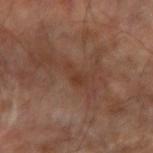<record>
  <biopsy_status>not biopsied; imaged during a skin examination</biopsy_status>
  <lighting>cross-polarized</lighting>
  <image>
    <source>total-body photography crop</source>
    <field_of_view_mm>15</field_of_view_mm>
  </image>
  <site>right forearm</site>
  <automated_metrics>
    <area_mm2_approx>3.0</area_mm2_approx>
    <eccentricity>0.9</eccentricity>
    <shape_asymmetry>0.5</shape_asymmetry>
  </automated_metrics>
  <lesion_size>
    <long_diameter_mm_approx>3.0</long_diameter_mm_approx>
  </lesion_size>
</record>